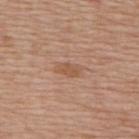| field | value |
|---|---|
| biopsy status | no biopsy performed (imaged during a skin exam) |
| lesion diameter | about 3 mm |
| location | the upper back |
| tile lighting | white-light illumination |
| subject | male, aged approximately 80 |
| image | total-body-photography crop, ~15 mm field of view |